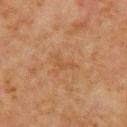No biopsy was performed on this lesion — it was imaged during a full skin examination and was not determined to be concerning. A roughly 15 mm field-of-view crop from a total-body skin photograph. A male subject roughly 70 years of age. The lesion's longest dimension is about 3 mm. Located on the chest. This is a cross-polarized tile.